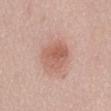The lesion was photographed on a routine skin check and not biopsied; there is no pathology result. Measured at roughly 4 mm in maximum diameter. The patient is a male aged 53 to 57. The lesion is located on the abdomen. A lesion tile, about 15 mm wide, cut from a 3D total-body photograph. Captured under white-light illumination. The total-body-photography lesion software estimated a lesion color around L≈59 a*≈23 b*≈28 in CIELAB and a lesion–skin lightness drop of about 9. The software also gave a color-variation rating of about 4/10 and peripheral color asymmetry of about 1.5. The software also gave a classifier nevus-likeness of about 85/100.